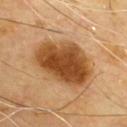  site: chest
  lighting: cross-polarized
  automated_metrics:
    area_mm2_approx: 29.0
    eccentricity: 0.75
    shape_asymmetry: 0.15
    cielab_L: 49
    cielab_a: 23
    cielab_b: 41
    vs_skin_darker_L: 17.0
    vs_skin_contrast_norm: 11.5
  patient:
    sex: male
    age_approx: 65
  image:
    source: total-body photography crop
    field_of_view_mm: 15
  lesion_size:
    long_diameter_mm_approx: 7.0